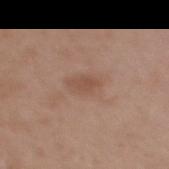Notes:
* workup — total-body-photography surveillance lesion; no biopsy
* site — the upper back
* automated lesion analysis — a footprint of about 5.5 mm², an outline eccentricity of about 0.8 (0 = round, 1 = elongated), and two-axis asymmetry of about 0.15; a mean CIELAB color near L≈51 a*≈19 b*≈28, a lesion–skin lightness drop of about 7, and a lesion-to-skin contrast of about 5.5 (normalized; higher = more distinct); a border-irregularity index near 2/10 and a within-lesion color-variation index near 2.5/10; a nevus-likeness score of about 0/100 and a lesion-detection confidence of about 100/100
* imaging modality — ~15 mm tile from a whole-body skin photo
* subject — female, roughly 40 years of age
* tile lighting — white-light illumination
* diameter — about 3.5 mm